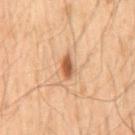<case>
  <biopsy_status>not biopsied; imaged during a skin examination</biopsy_status>
</case>